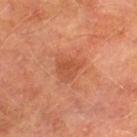Case summary:
• notes — total-body-photography surveillance lesion; no biopsy
• acquisition — ~15 mm crop, total-body skin-cancer survey
• illumination — cross-polarized illumination
• location — the leg
• lesion diameter — ~3 mm (longest diameter)
• subject — male, approximately 75 years of age